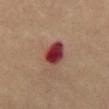This lesion was catalogued during total-body skin photography and was not selected for biopsy. Imaged with cross-polarized lighting. A close-up tile cropped from a whole-body skin photograph, about 15 mm across. A female subject, aged 53 to 57. Measured at roughly 3.5 mm in maximum diameter. The lesion is located on the abdomen.